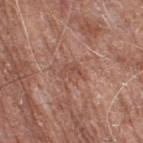A region of skin cropped from a whole-body photographic capture, roughly 15 mm wide. Imaged with white-light lighting. The patient is a male aged 78 to 82. Measured at roughly 3 mm in maximum diameter. On the leg.